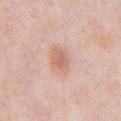This lesion was catalogued during total-body skin photography and was not selected for biopsy.